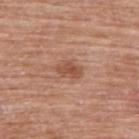Part of a total-body skin-imaging series; this lesion was reviewed on a skin check and was not flagged for biopsy. On the upper back. Approximately 3 mm at its widest. A lesion tile, about 15 mm wide, cut from a 3D total-body photograph. A female subject roughly 60 years of age.